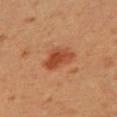Impression:
This lesion was catalogued during total-body skin photography and was not selected for biopsy.
Image and clinical context:
Cropped from a whole-body photographic skin survey; the tile spans about 15 mm. The patient is a female aged 28 to 32. Approximately 4.5 mm at its widest. The lesion is on the back. The lesion-visualizer software estimated a footprint of about 8.5 mm², an eccentricity of roughly 0.8, and a shape-asymmetry score of about 0.3 (0 = symmetric). And it measured a nevus-likeness score of about 95/100 and a lesion-detection confidence of about 100/100. The tile uses cross-polarized illumination.